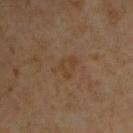The tile uses cross-polarized illumination.
The lesion is located on the chest.
Approximately 3 mm at its widest.
The total-body-photography lesion software estimated a footprint of about 5.5 mm², an outline eccentricity of about 0.7 (0 = round, 1 = elongated), and a shape-asymmetry score of about 0.35 (0 = symmetric). It also reported a classifier nevus-likeness of about 0/100 and lesion-presence confidence of about 100/100.
The patient is a male in their mid- to late 60s.
Cropped from a whole-body photographic skin survey; the tile spans about 15 mm.